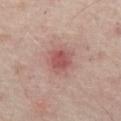Case summary:
- follow-up · catalogued during a skin exam; not biopsied
- location · the abdomen
- patient · aged approximately 55
- imaging modality · ~15 mm crop, total-body skin-cancer survey
- tile lighting · cross-polarized illumination
- TBP lesion metrics · a classifier nevus-likeness of about 0/100 and a detector confidence of about 100 out of 100 that the crop contains a lesion
- size · ~3 mm (longest diameter)